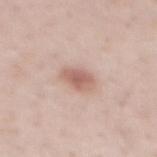  image:
    source: total-body photography crop
    field_of_view_mm: 15
  lighting: white-light
  patient:
    sex: male
    age_approx: 40
  lesion_size:
    long_diameter_mm_approx: 3.0
  site: mid back